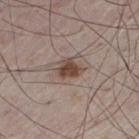acquisition — ~15 mm crop, total-body skin-cancer survey | automated metrics — a lesion area of about 6 mm², an eccentricity of roughly 0.7, and a symmetry-axis asymmetry near 0.25; a nevus-likeness score of about 90/100 and a lesion-detection confidence of about 100/100 | patient — male, in their mid- to late 70s | size — ≈3.5 mm | body site — the left thigh.